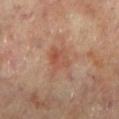Assessment:
Recorded during total-body skin imaging; not selected for excision or biopsy.
Acquisition and patient details:
The patient is a female in their mid- to late 60s. Measured at roughly 3.5 mm in maximum diameter. Captured under cross-polarized illumination. This image is a 15 mm lesion crop taken from a total-body photograph. From the right leg. The total-body-photography lesion software estimated a border-irregularity rating of about 3/10 and a within-lesion color-variation index near 4.5/10. And it measured a nevus-likeness score of about 0/100 and lesion-presence confidence of about 100/100.